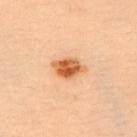- follow-up — imaged on a skin check; not biopsied
- tile lighting — cross-polarized
- acquisition — ~15 mm tile from a whole-body skin photo
- subject — female, about 40 years old
- lesion size — ≈4 mm
- location — the chest
- TBP lesion metrics — an area of roughly 7 mm²; an average lesion color of about L≈49 a*≈23 b*≈36 (CIELAB) and a lesion–skin lightness drop of about 13; a classifier nevus-likeness of about 100/100 and lesion-presence confidence of about 100/100